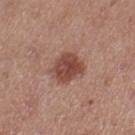<record>
  <biopsy_status>not biopsied; imaged during a skin examination</biopsy_status>
  <lesion_size>
    <long_diameter_mm_approx>3.5</long_diameter_mm_approx>
  </lesion_size>
  <site>left thigh</site>
  <patient>
    <sex>female</sex>
    <age_approx>40</age_approx>
  </patient>
  <image>
    <source>total-body photography crop</source>
    <field_of_view_mm>15</field_of_view_mm>
  </image>
</record>